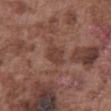No biopsy was performed on this lesion — it was imaged during a full skin examination and was not determined to be concerning. The lesion is on the abdomen. This is a white-light tile. Automated tile analysis of the lesion measured a footprint of about 4.5 mm², an eccentricity of roughly 0.7, and a shape-asymmetry score of about 0.2 (0 = symmetric). The analysis additionally found a lesion color around L≈40 a*≈20 b*≈25 in CIELAB, about 8 CIELAB-L* units darker than the surrounding skin, and a lesion-to-skin contrast of about 6.5 (normalized; higher = more distinct). The subject is a male approximately 75 years of age. A close-up tile cropped from a whole-body skin photograph, about 15 mm across. Longest diameter approximately 3 mm.